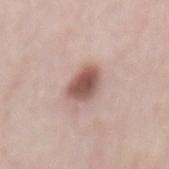automated metrics = an average lesion color of about L≈54 a*≈21 b*≈23 (CIELAB), roughly 16 lightness units darker than nearby skin, and a normalized border contrast of about 10; a within-lesion color-variation index near 3.5/10 and peripheral color asymmetry of about 1 | imaging modality = ~15 mm tile from a whole-body skin photo | patient = female, aged 28 to 32 | body site = the mid back | size = ~4 mm (longest diameter) | tile lighting = white-light illumination.